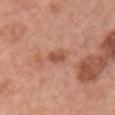Q: Was a biopsy performed?
A: no biopsy performed (imaged during a skin exam)
Q: Automated lesion metrics?
A: a footprint of about 3.5 mm², an eccentricity of roughly 0.75, and a symmetry-axis asymmetry near 0.15; a mean CIELAB color near L≈53 a*≈25 b*≈32, about 10 CIELAB-L* units darker than the surrounding skin, and a normalized lesion–skin contrast near 7; lesion-presence confidence of about 100/100
Q: Where on the body is the lesion?
A: the chest
Q: Patient demographics?
A: female, aged 58–62
Q: What kind of image is this?
A: 15 mm crop, total-body photography
Q: What is the lesion's diameter?
A: ~2.5 mm (longest diameter)